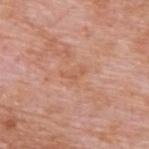biopsy status: imaged on a skin check; not biopsied | subject: male, aged 58–62 | body site: the upper back | automated metrics: a footprint of about 3 mm² and a shape eccentricity near 0.85; an average lesion color of about L≈59 a*≈24 b*≈34 (CIELAB), roughly 5 lightness units darker than nearby skin, and a lesion-to-skin contrast of about 4.5 (normalized; higher = more distinct); a border-irregularity rating of about 5.5/10 and a within-lesion color-variation index near 0/10; a detector confidence of about 100 out of 100 that the crop contains a lesion | lighting: white-light | acquisition: 15 mm crop, total-body photography | lesion size: ≈3 mm.